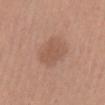Assessment: Part of a total-body skin-imaging series; this lesion was reviewed on a skin check and was not flagged for biopsy. Clinical summary: Captured under white-light illumination. Automated image analysis of the tile measured a footprint of about 10 mm². The software also gave about 7 CIELAB-L* units darker than the surrounding skin. The analysis additionally found a border-irregularity rating of about 2.5/10 and a peripheral color-asymmetry measure near 0.5. And it measured an automated nevus-likeness rating near 35 out of 100. A female patient aged approximately 30. A 15 mm close-up tile from a total-body photography series done for melanoma screening. Located on the abdomen.